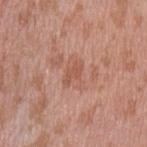biopsy status — no biopsy performed (imaged during a skin exam)
image-analysis metrics — an area of roughly 3 mm², an eccentricity of roughly 0.85, and two-axis asymmetry of about 0.4; a lesion–skin lightness drop of about 7 and a lesion-to-skin contrast of about 5.5 (normalized; higher = more distinct); radial color variation of about 0
anatomic site — the left upper arm
image source — total-body-photography crop, ~15 mm field of view
patient — male, aged around 40
size — about 2.5 mm